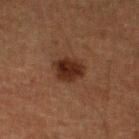Impression: The lesion was photographed on a routine skin check and not biopsied; there is no pathology result. Image and clinical context: A region of skin cropped from a whole-body photographic capture, roughly 15 mm wide. The lesion is located on the left lower leg. The patient is a male in their mid-70s.